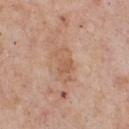Clinical impression: Part of a total-body skin-imaging series; this lesion was reviewed on a skin check and was not flagged for biopsy. Clinical summary: On the chest. A lesion tile, about 15 mm wide, cut from a 3D total-body photograph. Measured at roughly 4 mm in maximum diameter. A male patient aged 53–57. Imaged with white-light lighting.